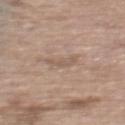This lesion was catalogued during total-body skin photography and was not selected for biopsy. The lesion is on the mid back. The lesion-visualizer software estimated an area of roughly 3 mm², a shape eccentricity near 0.9, and a symmetry-axis asymmetry near 0.55. The software also gave roughly 7 lightness units darker than nearby skin and a lesion-to-skin contrast of about 5 (normalized; higher = more distinct). The software also gave internal color variation of about 0 on a 0–10 scale. Captured under white-light illumination. Longest diameter approximately 3 mm. A female patient, aged around 65. A lesion tile, about 15 mm wide, cut from a 3D total-body photograph.